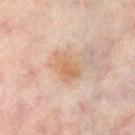Impression:
Part of a total-body skin-imaging series; this lesion was reviewed on a skin check and was not flagged for biopsy.
Image and clinical context:
This image is a 15 mm lesion crop taken from a total-body photograph. The patient is a female about 50 years old. Imaged with cross-polarized lighting. The lesion is located on the left lower leg.